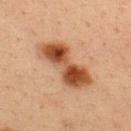{
  "biopsy_status": "not biopsied; imaged during a skin examination",
  "lighting": "cross-polarized",
  "site": "upper back",
  "patient": {
    "sex": "male",
    "age_approx": 35
  },
  "image": {
    "source": "total-body photography crop",
    "field_of_view_mm": 15
  }
}